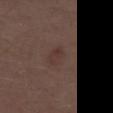The lesion was tiled from a total-body skin photograph and was not biopsied. The lesion is on the right thigh. The patient is a male approximately 70 years of age. Cropped from a total-body skin-imaging series; the visible field is about 15 mm.